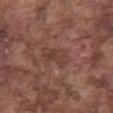follow-up = total-body-photography surveillance lesion; no biopsy
subject = male, roughly 75 years of age
illumination = white-light illumination
image source = ~15 mm tile from a whole-body skin photo
body site = the abdomen
lesion diameter = ≈4 mm